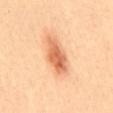The lesion was tiled from a total-body skin photograph and was not biopsied. The recorded lesion diameter is about 5.5 mm. The patient is a female aged 33–37. Captured under cross-polarized illumination. The lesion is on the mid back. A 15 mm close-up tile from a total-body photography series done for melanoma screening.